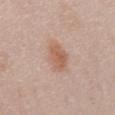Imaged during a routine full-body skin examination; the lesion was not biopsied and no histopathology is available. Cropped from a total-body skin-imaging series; the visible field is about 15 mm. Located on the mid back. The patient is a male aged 53–57.